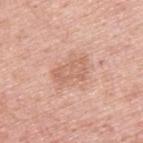The lesion was tiled from a total-body skin photograph and was not biopsied. The lesion is located on the back. A male subject, aged around 50. A 15 mm close-up tile from a total-body photography series done for melanoma screening.